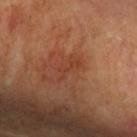{
  "biopsy_status": "not biopsied; imaged during a skin examination",
  "patient": {
    "sex": "female",
    "age_approx": 55
  },
  "lighting": "cross-polarized",
  "site": "head or neck",
  "image": {
    "source": "total-body photography crop",
    "field_of_view_mm": 15
  },
  "automated_metrics": {
    "area_mm2_approx": 3.0,
    "eccentricity": 0.9,
    "shape_asymmetry": 0.5,
    "border_irregularity_0_10": 6.5,
    "color_variation_0_10": 0.0,
    "peripheral_color_asymmetry": 0.0
  }
}